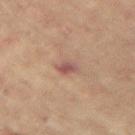Q: Is there a histopathology result?
A: catalogued during a skin exam; not biopsied
Q: Where on the body is the lesion?
A: the left arm
Q: What are the patient's age and sex?
A: female, approximately 80 years of age
Q: What kind of image is this?
A: ~15 mm tile from a whole-body skin photo